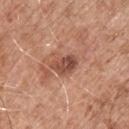Captured during whole-body skin photography for melanoma surveillance; the lesion was not biopsied.
Automated image analysis of the tile measured an area of roughly 5.5 mm², an outline eccentricity of about 0.8 (0 = round, 1 = elongated), and two-axis asymmetry of about 0.25. The software also gave a lesion color around L≈48 a*≈24 b*≈29 in CIELAB, roughly 13 lightness units darker than nearby skin, and a lesion-to-skin contrast of about 9 (normalized; higher = more distinct). It also reported a within-lesion color-variation index near 5.5/10 and a peripheral color-asymmetry measure near 2. The analysis additionally found a nevus-likeness score of about 5/100.
A 15 mm close-up extracted from a 3D total-body photography capture.
Measured at roughly 3.5 mm in maximum diameter.
Located on the chest.
Imaged with white-light lighting.
A male subject, aged approximately 55.